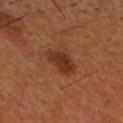Recorded during total-body skin imaging; not selected for excision or biopsy. Cropped from a whole-body photographic skin survey; the tile spans about 15 mm. The lesion is on the head or neck. A male patient, approximately 60 years of age. Imaged with cross-polarized lighting. The lesion-visualizer software estimated an average lesion color of about L≈24 a*≈19 b*≈25 (CIELAB), a lesion–skin lightness drop of about 8, and a normalized border contrast of about 8.5. The software also gave radial color variation of about 1. Measured at roughly 3.5 mm in maximum diameter.